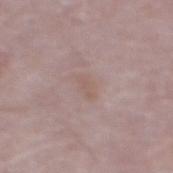Recorded during total-body skin imaging; not selected for excision or biopsy.
A 15 mm crop from a total-body photograph taken for skin-cancer surveillance.
Captured under white-light illumination.
The lesion is located on the abdomen.
About 3 mm across.
The patient is a male aged around 75.